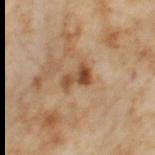Recorded during total-body skin imaging; not selected for excision or biopsy. The patient is a female about 55 years old. The lesion's longest dimension is about 3.5 mm. The lesion is on the left thigh. A region of skin cropped from a whole-body photographic capture, roughly 15 mm wide.